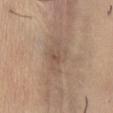notes — total-body-photography surveillance lesion; no biopsy
anatomic site — the abdomen
illumination — white-light
size — ≈5 mm
acquisition — total-body-photography crop, ~15 mm field of view
patient — female, roughly 45 years of age